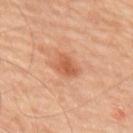Part of a total-body skin-imaging series; this lesion was reviewed on a skin check and was not flagged for biopsy.
A male subject, about 65 years old.
A close-up tile cropped from a whole-body skin photograph, about 15 mm across.
The tile uses cross-polarized illumination.
Longest diameter approximately 3 mm.
From the back.